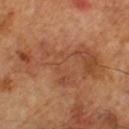{
  "biopsy_status": "not biopsied; imaged during a skin examination",
  "patient": {
    "sex": "male",
    "age_approx": 65
  },
  "image": {
    "source": "total-body photography crop",
    "field_of_view_mm": 15
  }
}